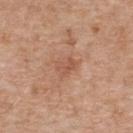The lesion was tiled from a total-body skin photograph and was not biopsied.
The subject is a female roughly 75 years of age.
Located on the back.
Captured under white-light illumination.
A lesion tile, about 15 mm wide, cut from a 3D total-body photograph.
Measured at roughly 2.5 mm in maximum diameter.